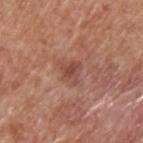The lesion was tiled from a total-body skin photograph and was not biopsied. A male patient about 65 years old. A region of skin cropped from a whole-body photographic capture, roughly 15 mm wide. Located on the upper back. The lesion's longest dimension is about 2.5 mm. Imaged with white-light lighting.